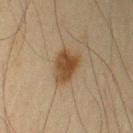{"biopsy_status": "not biopsied; imaged during a skin examination", "lesion_size": {"long_diameter_mm_approx": 4.0}, "site": "left upper arm", "image": {"source": "total-body photography crop", "field_of_view_mm": 15}, "lighting": "cross-polarized", "patient": {"sex": "male", "age_approx": 65}}